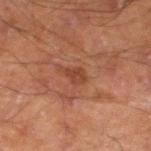Q: Was a biopsy performed?
A: catalogued during a skin exam; not biopsied
Q: Patient demographics?
A: male, about 70 years old
Q: Lesion size?
A: ~3 mm (longest diameter)
Q: Automated lesion metrics?
A: an area of roughly 3.5 mm² and a shape-asymmetry score of about 0.4 (0 = symmetric); an average lesion color of about L≈35 a*≈22 b*≈27 (CIELAB) and a lesion-to-skin contrast of about 6 (normalized; higher = more distinct); a border-irregularity index near 4/10, a within-lesion color-variation index near 1.5/10, and peripheral color asymmetry of about 0.5; an automated nevus-likeness rating near 0 out of 100
Q: Illumination type?
A: cross-polarized
Q: What is the imaging modality?
A: total-body-photography crop, ~15 mm field of view
Q: Where on the body is the lesion?
A: the left lower leg